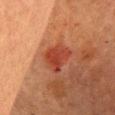This lesion was catalogued during total-body skin photography and was not selected for biopsy.
The total-body-photography lesion software estimated a lesion area of about 9 mm², an eccentricity of roughly 0.45, and a symmetry-axis asymmetry near 0.3. It also reported a lesion color around L≈40 a*≈31 b*≈33 in CIELAB, a lesion–skin lightness drop of about 8, and a normalized lesion–skin contrast near 7. It also reported a border-irregularity index near 3/10, a color-variation rating of about 3.5/10, and peripheral color asymmetry of about 1. And it measured an automated nevus-likeness rating near 90 out of 100.
The lesion's longest dimension is about 3.5 mm.
The tile uses cross-polarized illumination.
A female patient, aged approximately 60.
A close-up tile cropped from a whole-body skin photograph, about 15 mm across.
From the front of the torso.